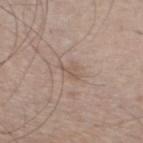• notes: no biopsy performed (imaged during a skin exam)
• acquisition: total-body-photography crop, ~15 mm field of view
• automated lesion analysis: a footprint of about 4 mm², a shape eccentricity near 0.7, and a symmetry-axis asymmetry near 0.4
• site: the left lower leg
• tile lighting: white-light illumination
• subject: male, aged approximately 60
• lesion diameter: about 3 mm